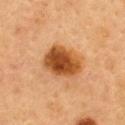follow-up: catalogued during a skin exam; not biopsied | image: ~15 mm tile from a whole-body skin photo | location: the upper back | patient: female, in their 60s.